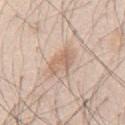Clinical impression:
Part of a total-body skin-imaging series; this lesion was reviewed on a skin check and was not flagged for biopsy.
Image and clinical context:
From the mid back. A 15 mm crop from a total-body photograph taken for skin-cancer surveillance. The tile uses white-light illumination. About 4 mm across. A male subject aged 43 to 47.